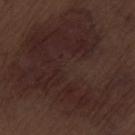The lesion was photographed on a routine skin check and not biopsied; there is no pathology result. On the leg. The lesion-visualizer software estimated a lesion area of about 100 mm² and a shape-asymmetry score of about 0.6 (0 = symmetric). The software also gave border irregularity of about 9.5 on a 0–10 scale and a peripheral color-asymmetry measure near 1. The software also gave a lesion-detection confidence of about 95/100. A male subject about 70 years old. About 14 mm across. This is a white-light tile. A 15 mm close-up extracted from a 3D total-body photography capture.